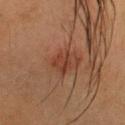Impression:
Captured during whole-body skin photography for melanoma surveillance; the lesion was not biopsied.
Clinical summary:
The lesion is located on the head or neck. This is a cross-polarized tile. About 3 mm across. A region of skin cropped from a whole-body photographic capture, roughly 15 mm wide. A female subject aged 38–42.